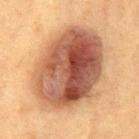acquisition — 15 mm crop, total-body photography | subject — female, aged 38–42 | automated metrics — an eccentricity of roughly 0.7 and two-axis asymmetry of about 0.1; a border-irregularity index near 1.5/10 and peripheral color asymmetry of about 4 | tile lighting — cross-polarized illumination | size — ~10 mm (longest diameter) | location — the mid back.